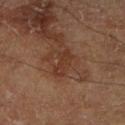Captured during whole-body skin photography for melanoma surveillance; the lesion was not biopsied.
Captured under cross-polarized illumination.
The total-body-photography lesion software estimated an area of roughly 4.5 mm², an outline eccentricity of about 0.9 (0 = round, 1 = elongated), and a symmetry-axis asymmetry near 0.4. The analysis additionally found a classifier nevus-likeness of about 0/100 and a lesion-detection confidence of about 100/100.
A 15 mm close-up tile from a total-body photography series done for melanoma screening.
A male patient aged 68–72.
The recorded lesion diameter is about 3.5 mm.
On the right lower leg.